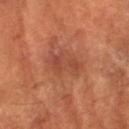- biopsy status — imaged on a skin check; not biopsied
- tile lighting — cross-polarized
- anatomic site — the arm
- automated metrics — roughly 5 lightness units darker than nearby skin and a normalized border contrast of about 5.5; a border-irregularity index near 5/10 and radial color variation of about 0.5; an automated nevus-likeness rating near 0 out of 100 and a lesion-detection confidence of about 100/100
- patient — female, approximately 80 years of age
- diameter — ≈4 mm
- image source — ~15 mm crop, total-body skin-cancer survey